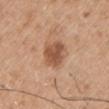Assessment: Recorded during total-body skin imaging; not selected for excision or biopsy. Background: From the right upper arm. A close-up tile cropped from a whole-body skin photograph, about 15 mm across. This is a white-light tile. Automated image analysis of the tile measured border irregularity of about 2 on a 0–10 scale, a within-lesion color-variation index near 3/10, and a peripheral color-asymmetry measure near 1. The software also gave a nevus-likeness score of about 65/100 and a detector confidence of about 100 out of 100 that the crop contains a lesion. A male subject, about 50 years old.